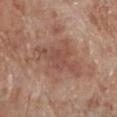No biopsy was performed on this lesion — it was imaged during a full skin examination and was not determined to be concerning. The lesion's longest dimension is about 7 mm. The tile uses white-light illumination. An algorithmic analysis of the crop reported a footprint of about 18 mm², an outline eccentricity of about 0.85 (0 = round, 1 = elongated), and a shape-asymmetry score of about 0.35 (0 = symmetric). The software also gave an average lesion color of about L≈50 a*≈21 b*≈27 (CIELAB) and about 9 CIELAB-L* units darker than the surrounding skin. It also reported an automated nevus-likeness rating near 0 out of 100 and a detector confidence of about 100 out of 100 that the crop contains a lesion. A 15 mm close-up extracted from a 3D total-body photography capture. A male patient aged around 70. Located on the left lower leg.